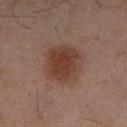Findings:
• notes: no biopsy performed (imaged during a skin exam)
• location: the left lower leg
• image source: 15 mm crop, total-body photography
• automated lesion analysis: a mean CIELAB color near L≈30 a*≈16 b*≈21, a lesion–skin lightness drop of about 8, and a normalized lesion–skin contrast near 8.5
• subject: male, aged around 45
• lighting: cross-polarized illumination
• size: ~5 mm (longest diameter)